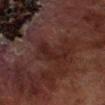The lesion was tiled from a total-body skin photograph and was not biopsied. Measured at roughly 4 mm in maximum diameter. This image is a 15 mm lesion crop taken from a total-body photograph. A male patient, in their 70s. Imaged with cross-polarized lighting. The lesion is on the right lower leg.